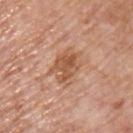Q: How was this image acquired?
A: total-body-photography crop, ~15 mm field of view
Q: Where on the body is the lesion?
A: the chest
Q: Who is the patient?
A: male, aged around 75